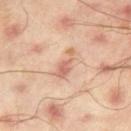<lesion>
<biopsy_status>not biopsied; imaged during a skin examination</biopsy_status>
<lighting>cross-polarized</lighting>
<site>right thigh</site>
<image>
  <source>total-body photography crop</source>
  <field_of_view_mm>15</field_of_view_mm>
</image>
<patient>
  <sex>male</sex>
  <age_approx>45</age_approx>
</patient>
<lesion_size>
  <long_diameter_mm_approx>4.0</long_diameter_mm_approx>
</lesion_size>
<automated_metrics>
  <border_irregularity_0_10>6.0</border_irregularity_0_10>
  <color_variation_0_10>1.5</color_variation_0_10>
  <peripheral_color_asymmetry>0.5</peripheral_color_asymmetry>
  <lesion_detection_confidence_0_100>100</lesion_detection_confidence_0_100>
</automated_metrics>
</lesion>